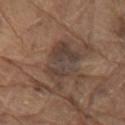notes = total-body-photography surveillance lesion; no biopsy | patient = male, aged 78 to 82 | body site = the right upper arm | image = 15 mm crop, total-body photography.